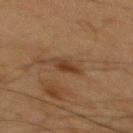Impression:
Imaged during a routine full-body skin examination; the lesion was not biopsied and no histopathology is available.
Acquisition and patient details:
A lesion tile, about 15 mm wide, cut from a 3D total-body photograph. Imaged with cross-polarized lighting. The lesion is on the mid back. Measured at roughly 3 mm in maximum diameter. A male subject roughly 65 years of age.